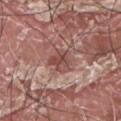Impression: No biopsy was performed on this lesion — it was imaged during a full skin examination and was not determined to be concerning. Background: This image is a 15 mm lesion crop taken from a total-body photograph. A male subject, aged approximately 40. The lesion is located on the upper back. Measured at roughly 3.5 mm in maximum diameter. This is a white-light tile.